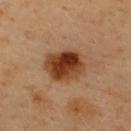Clinical impression: This lesion was catalogued during total-body skin photography and was not selected for biopsy. Background: A male subject, about 40 years old. The lesion-visualizer software estimated a symmetry-axis asymmetry near 0.1. It also reported a mean CIELAB color near L≈40 a*≈24 b*≈35, roughly 16 lightness units darker than nearby skin, and a lesion-to-skin contrast of about 12.5 (normalized; higher = more distinct). The analysis additionally found a nevus-likeness score of about 100/100 and a detector confidence of about 100 out of 100 that the crop contains a lesion. Cropped from a total-body skin-imaging series; the visible field is about 15 mm. From the upper back.